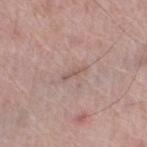notes — total-body-photography surveillance lesion; no biopsy
location — the left lower leg
subject — male, roughly 70 years of age
tile lighting — white-light
image source — total-body-photography crop, ~15 mm field of view